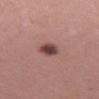<case>
<biopsy_status>not biopsied; imaged during a skin examination</biopsy_status>
<lesion_size>
  <long_diameter_mm_approx>3.0</long_diameter_mm_approx>
</lesion_size>
<site>right thigh</site>
<lighting>white-light</lighting>
<image>
  <source>total-body photography crop</source>
  <field_of_view_mm>15</field_of_view_mm>
</image>
<patient>
  <sex>female</sex>
  <age_approx>40</age_approx>
</patient>
<automated_metrics>
  <cielab_L>44</cielab_L>
  <cielab_a>21</cielab_a>
  <cielab_b>21</cielab_b>
  <border_irregularity_0_10>2.0</border_irregularity_0_10>
  <color_variation_0_10>4.0</color_variation_0_10>
  <peripheral_color_asymmetry>1.0</peripheral_color_asymmetry>
</automated_metrics>
</case>